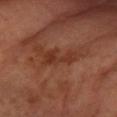Q: Is there a histopathology result?
A: total-body-photography surveillance lesion; no biopsy